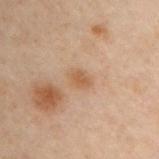{
  "biopsy_status": "not biopsied; imaged during a skin examination",
  "patient": {
    "sex": "male",
    "age_approx": 50
  },
  "automated_metrics": {
    "cielab_L": 47,
    "cielab_a": 16,
    "cielab_b": 29,
    "vs_skin_darker_L": 7.0,
    "vs_skin_contrast_norm": 6.0
  },
  "lesion_size": {
    "long_diameter_mm_approx": 3.0
  },
  "image": {
    "source": "total-body photography crop",
    "field_of_view_mm": 15
  },
  "site": "front of the torso"
}